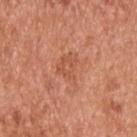Captured during whole-body skin photography for melanoma surveillance; the lesion was not biopsied. A 15 mm close-up extracted from a 3D total-body photography capture. The lesion-visualizer software estimated an area of roughly 7.5 mm² and an outline eccentricity of about 0.75 (0 = round, 1 = elongated). The patient is a male aged approximately 55. This is a white-light tile. From the upper back. Measured at roughly 4.5 mm in maximum diameter.